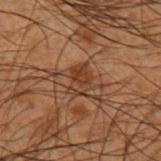tile lighting = cross-polarized illumination
lesion size = ~3 mm (longest diameter)
acquisition = 15 mm crop, total-body photography
site = the arm
subject = male, aged approximately 50